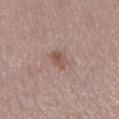follow-up: no biopsy performed (imaged during a skin exam) | location: the left lower leg | lesion size: ≈2.5 mm | illumination: white-light illumination | acquisition: total-body-photography crop, ~15 mm field of view | automated lesion analysis: an average lesion color of about L≈53 a*≈18 b*≈24 (CIELAB), a lesion–skin lightness drop of about 8, and a lesion-to-skin contrast of about 6 (normalized; higher = more distinct) | subject: female, aged 48 to 52.